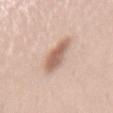notes: imaged on a skin check; not biopsied.